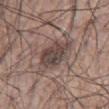Assessment:
The lesion was photographed on a routine skin check and not biopsied; there is no pathology result.
Acquisition and patient details:
A male patient, approximately 55 years of age. This is a white-light tile. A roughly 15 mm field-of-view crop from a total-body skin photograph. Located on the right upper arm. The recorded lesion diameter is about 4 mm.